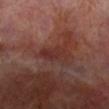follow-up = total-body-photography surveillance lesion; no biopsy | tile lighting = cross-polarized illumination | lesion diameter = about 4 mm | image source = ~15 mm crop, total-body skin-cancer survey | body site = the right lower leg | subject = male, in their 70s.